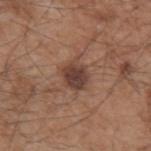Clinical impression:
The lesion was photographed on a routine skin check and not biopsied; there is no pathology result.
Acquisition and patient details:
A male patient in their mid-50s. Captured under white-light illumination. An algorithmic analysis of the crop reported a footprint of about 7 mm², an outline eccentricity of about 0.55 (0 = round, 1 = elongated), and a shape-asymmetry score of about 0.25 (0 = symmetric). It also reported a lesion-detection confidence of about 100/100. From the left upper arm. About 3.5 mm across. Cropped from a whole-body photographic skin survey; the tile spans about 15 mm.